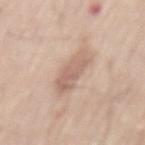Part of a total-body skin-imaging series; this lesion was reviewed on a skin check and was not flagged for biopsy. Automated tile analysis of the lesion measured a lesion area of about 9 mm², a shape eccentricity near 0.9, and a symmetry-axis asymmetry near 0.25. And it measured a lesion–skin lightness drop of about 10 and a lesion-to-skin contrast of about 6.5 (normalized; higher = more distinct). The software also gave border irregularity of about 3.5 on a 0–10 scale and radial color variation of about 1. And it measured a detector confidence of about 100 out of 100 that the crop contains a lesion. Longest diameter approximately 5 mm. The patient is a male in their mid- to late 60s. This image is a 15 mm lesion crop taken from a total-body photograph. The lesion is located on the back. Imaged with white-light lighting.